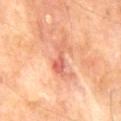No biopsy was performed on this lesion — it was imaged during a full skin examination and was not determined to be concerning. A region of skin cropped from a whole-body photographic capture, roughly 15 mm wide. The lesion is on the mid back. The lesion's longest dimension is about 4.5 mm. A male patient in their 70s.